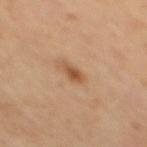biopsy_status: not biopsied; imaged during a skin examination
site: mid back
lighting: cross-polarized
image:
  source: total-body photography crop
  field_of_view_mm: 15
patient:
  sex: male
  age_approx: 60
lesion_size:
  long_diameter_mm_approx: 2.0